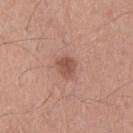Clinical summary: This is a white-light tile. Automated tile analysis of the lesion measured a footprint of about 5 mm² and an eccentricity of roughly 0.55. And it measured a within-lesion color-variation index near 3/10 and peripheral color asymmetry of about 1. The analysis additionally found a nevus-likeness score of about 75/100 and a lesion-detection confidence of about 100/100. A male subject, in their mid-50s. Located on the chest. A 15 mm crop from a total-body photograph taken for skin-cancer surveillance.